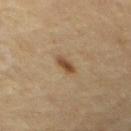No biopsy was performed on this lesion — it was imaged during a full skin examination and was not determined to be concerning. A close-up tile cropped from a whole-body skin photograph, about 15 mm across. The lesion is located on the chest. A male subject in their mid- to late 60s.